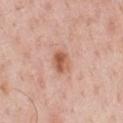The lesion was photographed on a routine skin check and not biopsied; there is no pathology result. Located on the chest. A male patient, aged 48–52. A region of skin cropped from a whole-body photographic capture, roughly 15 mm wide.